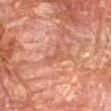On the right forearm. Captured under cross-polarized illumination. A region of skin cropped from a whole-body photographic capture, roughly 15 mm wide. A male subject, aged 78 to 82. Approximately 3.5 mm at its widest.